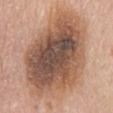Clinical impression:
Part of a total-body skin-imaging series; this lesion was reviewed on a skin check and was not flagged for biopsy.
Background:
A female patient about 65 years old. A 15 mm close-up extracted from a 3D total-body photography capture. The lesion-visualizer software estimated an average lesion color of about L≈53 a*≈19 b*≈29 (CIELAB), a lesion–skin lightness drop of about 16, and a normalized lesion–skin contrast near 10.5. Measured at roughly 13 mm in maximum diameter. The lesion is located on the chest.